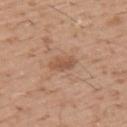location = the upper back
size = ~3.5 mm (longest diameter)
image = ~15 mm crop, total-body skin-cancer survey
illumination = white-light
patient = male, aged approximately 70